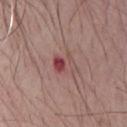  biopsy_status: not biopsied; imaged during a skin examination
  image:
    source: total-body photography crop
    field_of_view_mm: 15
  site: front of the torso
  patient:
    sex: male
    age_approx: 65